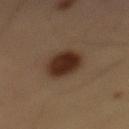Captured during whole-body skin photography for melanoma surveillance; the lesion was not biopsied.
A male patient in their mid-50s.
The lesion is located on the mid back.
Cropped from a whole-body photographic skin survey; the tile spans about 15 mm.